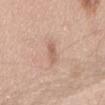Q: Was a biopsy performed?
A: total-body-photography surveillance lesion; no biopsy
Q: How was this image acquired?
A: 15 mm crop, total-body photography
Q: Where on the body is the lesion?
A: the abdomen
Q: Who is the patient?
A: male, approximately 30 years of age
Q: How large is the lesion?
A: ~3 mm (longest diameter)
Q: How was the tile lit?
A: white-light illumination